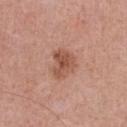Part of a total-body skin-imaging series; this lesion was reviewed on a skin check and was not flagged for biopsy.
The lesion is located on the front of the torso.
A male patient aged 53–57.
A 15 mm crop from a total-body photograph taken for skin-cancer surveillance.